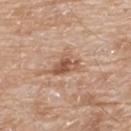| key | value |
|---|---|
| notes | catalogued during a skin exam; not biopsied |
| location | the back |
| image source | 15 mm crop, total-body photography |
| patient | male, approximately 80 years of age |